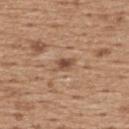follow-up: total-body-photography surveillance lesion; no biopsy
lesion diameter: ≈2.5 mm
image-analysis metrics: a lesion area of about 3.5 mm² and an outline eccentricity of about 0.8 (0 = round, 1 = elongated)
tile lighting: white-light
location: the back
image: 15 mm crop, total-body photography
patient: male, aged 63–67